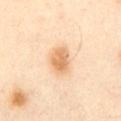| field | value |
|---|---|
| follow-up | total-body-photography surveillance lesion; no biopsy |
| illumination | cross-polarized |
| lesion diameter | ~3.5 mm (longest diameter) |
| site | the front of the torso |
| image | ~15 mm crop, total-body skin-cancer survey |
| subject | male, aged 53–57 |